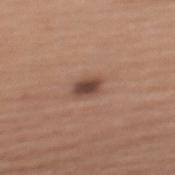On the upper back. Measured at roughly 3 mm in maximum diameter. A female patient aged 53–57. This is a white-light tile. Cropped from a whole-body photographic skin survey; the tile spans about 15 mm.